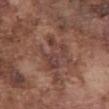The recorded lesion diameter is about 4.5 mm. The lesion is on the front of the torso. A male patient aged around 75. A 15 mm crop from a total-body photograph taken for skin-cancer surveillance. Automated tile analysis of the lesion measured a lesion area of about 10 mm² and an outline eccentricity of about 0.75 (0 = round, 1 = elongated). It also reported radial color variation of about 1.5.